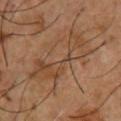{"biopsy_status": "not biopsied; imaged during a skin examination", "image": {"source": "total-body photography crop", "field_of_view_mm": 15}, "patient": {"sex": "male", "age_approx": 55}, "lighting": "cross-polarized", "automated_metrics": {"area_mm2_approx": 16.0, "eccentricity": 0.95, "shape_asymmetry": 0.6, "cielab_L": 43, "cielab_a": 18, "cielab_b": 31, "vs_skin_darker_L": 6.0, "vs_skin_contrast_norm": 5.0, "border_irregularity_0_10": 9.5, "color_variation_0_10": 5.0, "peripheral_color_asymmetry": 1.5, "nevus_likeness_0_100": 0, "lesion_detection_confidence_0_100": 80}, "site": "front of the torso", "lesion_size": {"long_diameter_mm_approx": 8.5}}